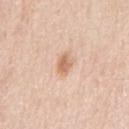Case summary:
– notes — total-body-photography surveillance lesion; no biopsy
– patient — male, approximately 50 years of age
– illumination — white-light
– lesion size — ≈2.5 mm
– body site — the right upper arm
– TBP lesion metrics — a footprint of about 3.5 mm² and two-axis asymmetry of about 0.2; a mean CIELAB color near L≈66 a*≈20 b*≈33, roughly 11 lightness units darker than nearby skin, and a normalized lesion–skin contrast near 7.5; an automated nevus-likeness rating near 65 out of 100 and a detector confidence of about 100 out of 100 that the crop contains a lesion
– acquisition — 15 mm crop, total-body photography